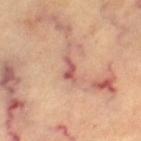| feature | finding |
|---|---|
| image | total-body-photography crop, ~15 mm field of view |
| body site | the left thigh |
| automated metrics | a lesion area of about 4 mm², a shape eccentricity near 0.9, and two-axis asymmetry of about 0.4; border irregularity of about 4.5 on a 0–10 scale, a color-variation rating of about 2.5/10, and peripheral color asymmetry of about 1 |
| patient | aged around 60 |